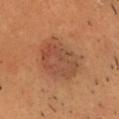This lesion was catalogued during total-body skin photography and was not selected for biopsy. The total-body-photography lesion software estimated an area of roughly 21 mm². It also reported a border-irregularity rating of about 2/10, a color-variation rating of about 5/10, and radial color variation of about 2. It also reported an automated nevus-likeness rating near 85 out of 100. A male subject, approximately 40 years of age. This image is a 15 mm lesion crop taken from a total-body photograph. On the head or neck. This is a cross-polarized tile.